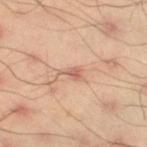Part of a total-body skin-imaging series; this lesion was reviewed on a skin check and was not flagged for biopsy.
The lesion is on the right thigh.
A male patient aged 43–47.
The tile uses cross-polarized illumination.
A 15 mm close-up extracted from a 3D total-body photography capture.
Automated tile analysis of the lesion measured a mean CIELAB color near L≈61 a*≈21 b*≈30, a lesion–skin lightness drop of about 10, and a lesion-to-skin contrast of about 6 (normalized; higher = more distinct). The analysis additionally found border irregularity of about 4.5 on a 0–10 scale and a color-variation rating of about 2.5/10. It also reported a lesion-detection confidence of about 100/100.